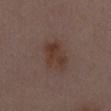The recorded lesion diameter is about 4 mm. A roughly 15 mm field-of-view crop from a total-body skin photograph. The tile uses white-light illumination. Automated tile analysis of the lesion measured a lesion area of about 9.5 mm², an outline eccentricity of about 0.8 (0 = round, 1 = elongated), and two-axis asymmetry of about 0.2. It also reported a border-irregularity rating of about 2.5/10, a within-lesion color-variation index near 3.5/10, and peripheral color asymmetry of about 1. A female patient aged approximately 30. Located on the chest.